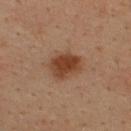Notes:
• biopsy status — no biopsy performed (imaged during a skin exam)
• automated lesion analysis — a nevus-likeness score of about 95/100 and a lesion-detection confidence of about 100/100
• subject — male, aged 33 to 37
• body site — the upper back
• acquisition — ~15 mm crop, total-body skin-cancer survey
• illumination — cross-polarized illumination